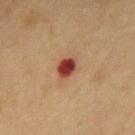{
  "biopsy_status": "not biopsied; imaged during a skin examination",
  "patient": {
    "sex": "male",
    "age_approx": 55
  },
  "lesion_size": {
    "long_diameter_mm_approx": 3.0
  },
  "image": {
    "source": "total-body photography crop",
    "field_of_view_mm": 15
  },
  "site": "back",
  "automated_metrics": {
    "area_mm2_approx": 5.0,
    "eccentricity": 0.7,
    "shape_asymmetry": 0.25,
    "cielab_L": 38,
    "cielab_a": 27,
    "cielab_b": 27,
    "vs_skin_darker_L": 16.0,
    "vs_skin_contrast_norm": 12.5,
    "border_irregularity_0_10": 2.0,
    "peripheral_color_asymmetry": 1.0
  }
}